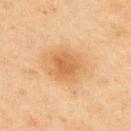Impression: Imaged during a routine full-body skin examination; the lesion was not biopsied and no histopathology is available. Image and clinical context: The subject is a male about 45 years old. Measured at roughly 3.5 mm in maximum diameter. The lesion-visualizer software estimated a lesion–skin lightness drop of about 7 and a normalized border contrast of about 6. It also reported a classifier nevus-likeness of about 65/100 and lesion-presence confidence of about 100/100. Captured under cross-polarized illumination. A lesion tile, about 15 mm wide, cut from a 3D total-body photograph. The lesion is located on the upper back.